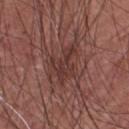| feature | finding |
|---|---|
| notes | no biopsy performed (imaged during a skin exam) |
| patient | male, aged approximately 65 |
| diameter | ~5.5 mm (longest diameter) |
| acquisition | ~15 mm crop, total-body skin-cancer survey |
| lighting | white-light |
| site | the chest |